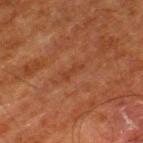Clinical impression:
Imaged during a routine full-body skin examination; the lesion was not biopsied and no histopathology is available.
Background:
Automated image analysis of the tile measured an average lesion color of about L≈31 a*≈22 b*≈28 (CIELAB) and a normalized border contrast of about 5. It also reported a color-variation rating of about 0/10 and a peripheral color-asymmetry measure near 0. The software also gave a classifier nevus-likeness of about 0/100 and a lesion-detection confidence of about 95/100. Longest diameter approximately 2.5 mm. Imaged with cross-polarized lighting. The subject is a male aged around 80. The lesion is on the left thigh. A 15 mm close-up tile from a total-body photography series done for melanoma screening.